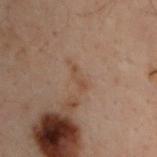The subject is a male approximately 30 years of age. On the upper back. This image is a 15 mm lesion crop taken from a total-body photograph.